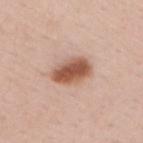| field | value |
|---|---|
| notes | no biopsy performed (imaged during a skin exam) |
| imaging modality | total-body-photography crop, ~15 mm field of view |
| subject | male, approximately 50 years of age |
| size | ≈4.5 mm |
| anatomic site | the back |
| TBP lesion metrics | a lesion area of about 10 mm², a shape eccentricity near 0.8, and two-axis asymmetry of about 0.15; an average lesion color of about L≈55 a*≈23 b*≈30 (CIELAB), about 16 CIELAB-L* units darker than the surrounding skin, and a normalized lesion–skin contrast near 10.5 |